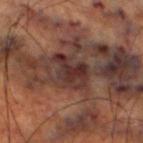The lesion was tiled from a total-body skin photograph and was not biopsied.
Measured at roughly 4.5 mm in maximum diameter.
Captured under cross-polarized illumination.
A 15 mm close-up tile from a total-body photography series done for melanoma screening.
A male patient in their 70s.
An algorithmic analysis of the crop reported border irregularity of about 4.5 on a 0–10 scale, internal color variation of about 5 on a 0–10 scale, and peripheral color asymmetry of about 1.5. And it measured a classifier nevus-likeness of about 0/100 and a lesion-detection confidence of about 65/100.
Located on the right thigh.